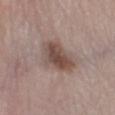Background: Automated tile analysis of the lesion measured a lesion area of about 14 mm², an eccentricity of roughly 0.8, and two-axis asymmetry of about 0.2. It also reported a mean CIELAB color near L≈48 a*≈16 b*≈22 and a lesion–skin lightness drop of about 11. It also reported a border-irregularity index near 2/10, internal color variation of about 5.5 on a 0–10 scale, and a peripheral color-asymmetry measure near 1.5. The analysis additionally found an automated nevus-likeness rating near 70 out of 100 and lesion-presence confidence of about 100/100. Imaged with white-light lighting. The subject is a female aged 53–57. Cropped from a whole-body photographic skin survey; the tile spans about 15 mm. From the left lower leg. Longest diameter approximately 5.5 mm.